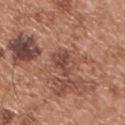No biopsy was performed on this lesion — it was imaged during a full skin examination and was not determined to be concerning. Cropped from a total-body skin-imaging series; the visible field is about 15 mm. A male patient, about 55 years old. Approximately 3.5 mm at its widest. On the chest. This is a white-light tile. Automated image analysis of the tile measured a border-irregularity rating of about 6/10 and radial color variation of about 1.